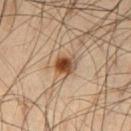Assessment:
The lesion was photographed on a routine skin check and not biopsied; there is no pathology result.
Image and clinical context:
A male patient in their mid- to late 40s. This is a cross-polarized tile. A close-up tile cropped from a whole-body skin photograph, about 15 mm across. On the left thigh. Automated image analysis of the tile measured a footprint of about 5 mm² and a symmetry-axis asymmetry near 0.15. And it measured border irregularity of about 1 on a 0–10 scale, a within-lesion color-variation index near 9/10, and peripheral color asymmetry of about 3.